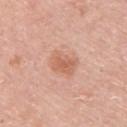- notes · catalogued during a skin exam; not biopsied
- patient · male, roughly 60 years of age
- size · about 3.5 mm
- imaging modality · ~15 mm crop, total-body skin-cancer survey
- anatomic site · the upper back